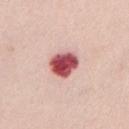Q: Was a biopsy performed?
A: catalogued during a skin exam; not biopsied
Q: What is the lesion's diameter?
A: ≈3.5 mm
Q: What did automated image analysis measure?
A: a footprint of about 9 mm², an outline eccentricity of about 0.4 (0 = round, 1 = elongated), and a symmetry-axis asymmetry near 0.15; border irregularity of about 1.5 on a 0–10 scale, internal color variation of about 5.5 on a 0–10 scale, and peripheral color asymmetry of about 1.5; lesion-presence confidence of about 100/100
Q: What lighting was used for the tile?
A: white-light
Q: Who is the patient?
A: female, aged 28 to 32
Q: What is the imaging modality?
A: total-body-photography crop, ~15 mm field of view
Q: Where on the body is the lesion?
A: the chest